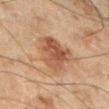Q: Was a biopsy performed?
A: imaged on a skin check; not biopsied
Q: What is the anatomic site?
A: the left lower leg
Q: What is the imaging modality?
A: ~15 mm tile from a whole-body skin photo
Q: Patient demographics?
A: male, aged 43 to 47